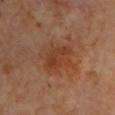| key | value |
|---|---|
| workup | catalogued during a skin exam; not biopsied |
| image-analysis metrics | an average lesion color of about L≈39 a*≈23 b*≈32 (CIELAB) and a normalized lesion–skin contrast near 6.5; a border-irregularity rating of about 3/10 and a peripheral color-asymmetry measure near 1.5 |
| acquisition | 15 mm crop, total-body photography |
| location | the front of the torso |
| lighting | cross-polarized |
| subject | female, in their mid- to late 60s |